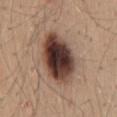No biopsy was performed on this lesion — it was imaged during a full skin examination and was not determined to be concerning. This image is a 15 mm lesion crop taken from a total-body photograph. Captured under white-light illumination. The subject is a female aged around 45. The lesion-visualizer software estimated a lesion area of about 23 mm², an eccentricity of roughly 0.8, and a symmetry-axis asymmetry near 0.1. And it measured a within-lesion color-variation index near 9.5/10 and peripheral color asymmetry of about 3. Located on the mid back.